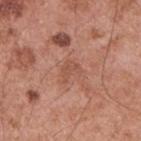Assessment: No biopsy was performed on this lesion — it was imaged during a full skin examination and was not determined to be concerning. Background: The patient is a male aged around 55. A 15 mm close-up extracted from a 3D total-body photography capture. On the upper back.